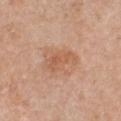Assessment:
Captured during whole-body skin photography for melanoma surveillance; the lesion was not biopsied.
Image and clinical context:
A region of skin cropped from a whole-body photographic capture, roughly 15 mm wide. Captured under white-light illumination. The subject is a female approximately 50 years of age. The lesion is on the chest.